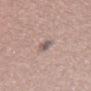The lesion was photographed on a routine skin check and not biopsied; there is no pathology result.
On the left leg.
A region of skin cropped from a whole-body photographic capture, roughly 15 mm wide.
The subject is a female in their mid- to late 30s.